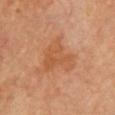| feature | finding |
|---|---|
| workup | no biopsy performed (imaged during a skin exam) |
| acquisition | ~15 mm crop, total-body skin-cancer survey |
| patient | female, aged approximately 70 |
| body site | the chest |